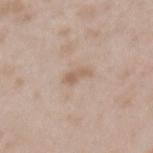Measured at roughly 2.5 mm in maximum diameter.
From the back.
The patient is a female aged around 30.
Captured under white-light illumination.
Cropped from a total-body skin-imaging series; the visible field is about 15 mm.